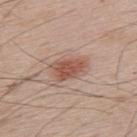  biopsy_status: not biopsied; imaged during a skin examination
  site: upper back
  lighting: white-light
  patient:
    sex: male
    age_approx: 65
  image:
    source: total-body photography crop
    field_of_view_mm: 15
  lesion_size:
    long_diameter_mm_approx: 4.5